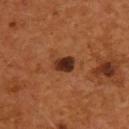Part of a total-body skin-imaging series; this lesion was reviewed on a skin check and was not flagged for biopsy.
From the back.
A male patient aged 53 to 57.
A 15 mm close-up tile from a total-body photography series done for melanoma screening.